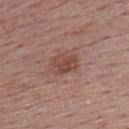| key | value |
|---|---|
| notes | no biopsy performed (imaged during a skin exam) |
| lighting | white-light |
| image source | 15 mm crop, total-body photography |
| site | the back |
| size | ≈3.5 mm |
| automated lesion analysis | a shape eccentricity near 0.7 and a symmetry-axis asymmetry near 0.15; a lesion color around L≈46 a*≈22 b*≈25 in CIELAB, a lesion–skin lightness drop of about 9, and a lesion-to-skin contrast of about 7 (normalized; higher = more distinct) |
| patient | female, aged approximately 55 |